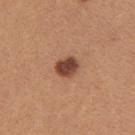workup=total-body-photography surveillance lesion; no biopsy | anatomic site=the left upper arm | tile lighting=white-light illumination | subject=female, roughly 25 years of age | acquisition=~15 mm crop, total-body skin-cancer survey | lesion diameter=~3 mm (longest diameter).